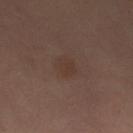Clinical impression:
Part of a total-body skin-imaging series; this lesion was reviewed on a skin check and was not flagged for biopsy.
Context:
On the left thigh. An algorithmic analysis of the crop reported a border-irregularity rating of about 2/10 and a peripheral color-asymmetry measure near 0.5. And it measured a nevus-likeness score of about 45/100 and a lesion-detection confidence of about 100/100. A female patient in their 50s. A 15 mm crop from a total-body photograph taken for skin-cancer surveillance. This is a cross-polarized tile.